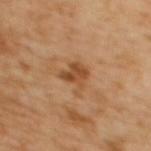body site — the upper back | image-analysis metrics — a lesion area of about 6.5 mm², an eccentricity of roughly 0.5, and a symmetry-axis asymmetry near 0.45; border irregularity of about 5.5 on a 0–10 scale and a peripheral color-asymmetry measure near 1; an automated nevus-likeness rating near 10 out of 100 and a lesion-detection confidence of about 100/100 | image source — total-body-photography crop, ~15 mm field of view | lesion diameter — about 3.5 mm | patient — female, approximately 55 years of age | tile lighting — cross-polarized illumination.